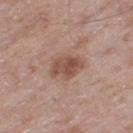{"biopsy_status": "not biopsied; imaged during a skin examination", "lighting": "white-light", "lesion_size": {"long_diameter_mm_approx": 4.0}, "site": "right thigh", "image": {"source": "total-body photography crop", "field_of_view_mm": 15}, "patient": {"sex": "male", "age_approx": 60}}